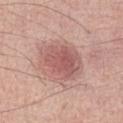{
  "biopsy_status": "not biopsied; imaged during a skin examination",
  "site": "abdomen",
  "image": {
    "source": "total-body photography crop",
    "field_of_view_mm": 15
  },
  "patient": {
    "sex": "male",
    "age_approx": 70
  }
}